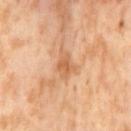Acquisition and patient details:
This image is a 15 mm lesion crop taken from a total-body photograph. The subject is a female about 55 years old. An algorithmic analysis of the crop reported roughly 9 lightness units darker than nearby skin. It also reported peripheral color asymmetry of about 0.5. The lesion is located on the left thigh.